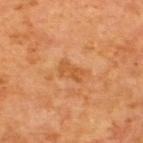Impression:
The lesion was tiled from a total-body skin photograph and was not biopsied.
Clinical summary:
Imaged with cross-polarized lighting. On the upper back. Automated tile analysis of the lesion measured a footprint of about 3.5 mm², an outline eccentricity of about 0.9 (0 = round, 1 = elongated), and a symmetry-axis asymmetry near 0.25. The software also gave a nevus-likeness score of about 0/100 and lesion-presence confidence of about 100/100. A male patient, approximately 65 years of age. The lesion's longest dimension is about 3 mm. A lesion tile, about 15 mm wide, cut from a 3D total-body photograph.